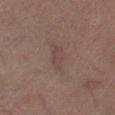Assessment:
This lesion was catalogued during total-body skin photography and was not selected for biopsy.
Background:
The subject is a male in their mid-60s. Located on the right lower leg. A region of skin cropped from a whole-body photographic capture, roughly 15 mm wide. Measured at roughly 3.5 mm in maximum diameter. Captured under cross-polarized illumination.